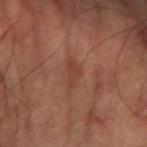{"lighting": "cross-polarized", "image": {"source": "total-body photography crop", "field_of_view_mm": 15}, "patient": {"sex": "male", "age_approx": 60}, "site": "left arm"}